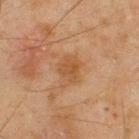Assessment: The lesion was photographed on a routine skin check and not biopsied; there is no pathology result. Background: Cropped from a total-body skin-imaging series; the visible field is about 15 mm. A male patient, aged approximately 45. Captured under cross-polarized illumination. Located on the upper back. The lesion's longest dimension is about 4 mm.